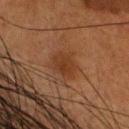Assessment: The lesion was photographed on a routine skin check and not biopsied; there is no pathology result. Clinical summary: Measured at roughly 3.5 mm in maximum diameter. An algorithmic analysis of the crop reported a lesion area of about 8 mm², an outline eccentricity of about 0.65 (0 = round, 1 = elongated), and two-axis asymmetry of about 0.2. The software also gave a border-irregularity index near 2/10, a color-variation rating of about 2/10, and radial color variation of about 0.5. On the head or neck. This image is a 15 mm lesion crop taken from a total-body photograph. The subject is a male approximately 60 years of age. Imaged with cross-polarized lighting.